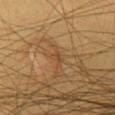Part of a total-body skin-imaging series; this lesion was reviewed on a skin check and was not flagged for biopsy.
A lesion tile, about 15 mm wide, cut from a 3D total-body photograph.
On the chest.
The total-body-photography lesion software estimated a lesion color around L≈48 a*≈18 b*≈36 in CIELAB, roughly 7 lightness units darker than nearby skin, and a normalized lesion–skin contrast near 5. And it measured a border-irregularity rating of about 6/10, a within-lesion color-variation index near 1/10, and a peripheral color-asymmetry measure near 0. It also reported an automated nevus-likeness rating near 0 out of 100 and lesion-presence confidence of about 0/100.
Captured under cross-polarized illumination.
A male patient, approximately 65 years of age.